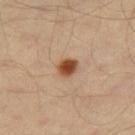– notes · catalogued during a skin exam; not biopsied
– size · about 2.5 mm
– patient · male, roughly 40 years of age
– automated lesion analysis · a lesion area of about 4.5 mm², a shape eccentricity near 0.5, and two-axis asymmetry of about 0.15; a border-irregularity rating of about 1.5/10, internal color variation of about 4 on a 0–10 scale, and radial color variation of about 1
– imaging modality · 15 mm crop, total-body photography
– anatomic site · the right thigh
– illumination · cross-polarized